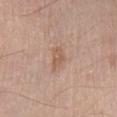Case summary:
- follow-up — total-body-photography surveillance lesion; no biopsy